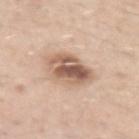The lesion was photographed on a routine skin check and not biopsied; there is no pathology result.
The lesion's longest dimension is about 4.5 mm.
A roughly 15 mm field-of-view crop from a total-body skin photograph.
On the left upper arm.
The subject is a male approximately 30 years of age.
Imaged with white-light lighting.